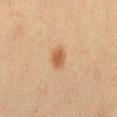Case summary:
* workup — total-body-photography surveillance lesion; no biopsy
* illumination — cross-polarized illumination
* automated metrics — a mean CIELAB color near L≈51 a*≈19 b*≈34 and a lesion-to-skin contrast of about 7.5 (normalized; higher = more distinct); border irregularity of about 1.5 on a 0–10 scale, a color-variation rating of about 2/10, and a peripheral color-asymmetry measure near 0.5; a classifier nevus-likeness of about 100/100 and a detector confidence of about 100 out of 100 that the crop contains a lesion
* patient — female, about 20 years old
* anatomic site — the left thigh
* size — ≈2.5 mm
* imaging modality — total-body-photography crop, ~15 mm field of view